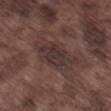A region of skin cropped from a whole-body photographic capture, roughly 15 mm wide. Longest diameter approximately 6.5 mm. A male subject, in their mid-70s. On the left thigh. This is a white-light tile. The total-body-photography lesion software estimated a footprint of about 16 mm² and a shape eccentricity near 0.85.